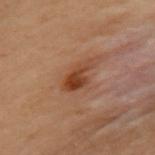Impression:
Recorded during total-body skin imaging; not selected for excision or biopsy.
Clinical summary:
The lesion is on the upper back. A 15 mm crop from a total-body photograph taken for skin-cancer surveillance. The patient is a female approximately 60 years of age.